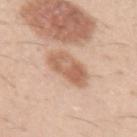Clinical impression:
This lesion was catalogued during total-body skin photography and was not selected for biopsy.
Image and clinical context:
A lesion tile, about 15 mm wide, cut from a 3D total-body photograph. The lesion-visualizer software estimated a lesion color around L≈62 a*≈21 b*≈32 in CIELAB and a normalized border contrast of about 7.5. It also reported a classifier nevus-likeness of about 0/100 and lesion-presence confidence of about 100/100. The lesion is on the left upper arm. The patient is a male aged approximately 30. This is a white-light tile.